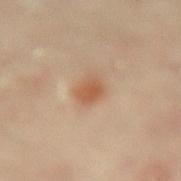This lesion was catalogued during total-body skin photography and was not selected for biopsy.
The lesion's longest dimension is about 2.5 mm.
The lesion is on the lower back.
A female subject, aged 58 to 62.
Cropped from a total-body skin-imaging series; the visible field is about 15 mm.
The tile uses cross-polarized illumination.